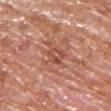Assessment:
The lesion was photographed on a routine skin check and not biopsied; there is no pathology result.
Context:
An algorithmic analysis of the crop reported a color-variation rating of about 5.5/10 and peripheral color asymmetry of about 2.5. It also reported a classifier nevus-likeness of about 0/100 and a lesion-detection confidence of about 80/100. From the front of the torso. The patient is a male in their mid- to late 60s. The lesion's longest dimension is about 3.5 mm. A region of skin cropped from a whole-body photographic capture, roughly 15 mm wide. Imaged with white-light lighting.